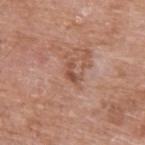follow-up: imaged on a skin check; not biopsied
illumination: white-light illumination
imaging modality: ~15 mm tile from a whole-body skin photo
body site: the left upper arm
patient: male, roughly 60 years of age
size: ≈3 mm